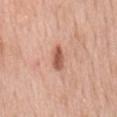Assessment: No biopsy was performed on this lesion — it was imaged during a full skin examination and was not determined to be concerning. Background: Captured under white-light illumination. A 15 mm close-up tile from a total-body photography series done for melanoma screening. The recorded lesion diameter is about 3.5 mm. On the mid back. A male patient roughly 60 years of age. The total-body-photography lesion software estimated a shape eccentricity near 0.85. And it measured a mean CIELAB color near L≈57 a*≈25 b*≈31. The analysis additionally found a border-irregularity rating of about 2.5/10, internal color variation of about 3 on a 0–10 scale, and radial color variation of about 1. The analysis additionally found a nevus-likeness score of about 90/100.